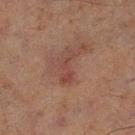Q: Is there a histopathology result?
A: imaged on a skin check; not biopsied